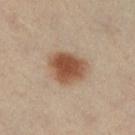No biopsy was performed on this lesion — it was imaged during a full skin examination and was not determined to be concerning. Imaged with cross-polarized lighting. From the left leg. Measured at roughly 4.5 mm in maximum diameter. A female subject aged around 40. A roughly 15 mm field-of-view crop from a total-body skin photograph.